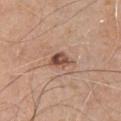Case summary:
* workup · catalogued during a skin exam; not biopsied
* site · the right upper arm
* subject · male, aged approximately 80
* image · 15 mm crop, total-body photography